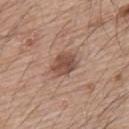biopsy status: no biopsy performed (imaged during a skin exam)
TBP lesion metrics: an average lesion color of about L≈49 a*≈20 b*≈26 (CIELAB) and a lesion-to-skin contrast of about 8.5 (normalized; higher = more distinct); an automated nevus-likeness rating near 50 out of 100 and lesion-presence confidence of about 100/100
size: ~3.5 mm (longest diameter)
tile lighting: white-light
acquisition: ~15 mm crop, total-body skin-cancer survey
anatomic site: the back
patient: male, roughly 65 years of age